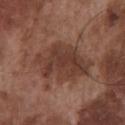Q: Is there a histopathology result?
A: imaged on a skin check; not biopsied
Q: Lesion location?
A: the chest
Q: What lighting was used for the tile?
A: white-light
Q: How large is the lesion?
A: about 7 mm
Q: What are the patient's age and sex?
A: male, aged 73–77
Q: What is the imaging modality?
A: total-body-photography crop, ~15 mm field of view